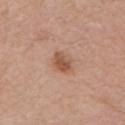Clinical impression: Captured during whole-body skin photography for melanoma surveillance; the lesion was not biopsied. Image and clinical context: The lesion is located on the left upper arm. The lesion-visualizer software estimated a lesion color around L≈54 a*≈22 b*≈31 in CIELAB, roughly 10 lightness units darker than nearby skin, and a normalized lesion–skin contrast near 7.5. The software also gave a within-lesion color-variation index near 3/10 and peripheral color asymmetry of about 1. A 15 mm crop from a total-body photograph taken for skin-cancer surveillance. About 3 mm across. Imaged with white-light lighting. The subject is a female aged 63–67.